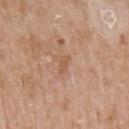acquisition = ~15 mm tile from a whole-body skin photo
subject = female, aged around 85 or older
body site = the left upper arm
size = ~2.5 mm (longest diameter)
image-analysis metrics = a mean CIELAB color near L≈56 a*≈20 b*≈33, roughly 7 lightness units darker than nearby skin, and a normalized lesion–skin contrast near 5.5
illumination = white-light illumination